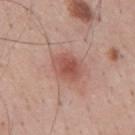{"image": {"source": "total-body photography crop", "field_of_view_mm": 15}, "site": "mid back", "patient": {"sex": "male", "age_approx": 35}, "automated_metrics": {"border_irregularity_0_10": 1.5, "color_variation_0_10": 3.5, "peripheral_color_asymmetry": 1.0}, "lighting": "white-light"}